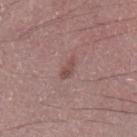notes — no biopsy performed (imaged during a skin exam)
subject — male, approximately 35 years of age
image source — 15 mm crop, total-body photography
size — ~2.5 mm (longest diameter)
body site — the left lower leg
illumination — white-light